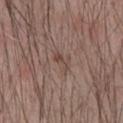Acquisition and patient details: On the right forearm. A male patient in their mid- to late 20s. Cropped from a total-body skin-imaging series; the visible field is about 15 mm.